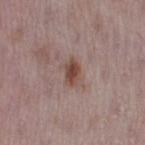{
  "biopsy_status": "not biopsied; imaged during a skin examination",
  "lesion_size": {
    "long_diameter_mm_approx": 3.0
  },
  "image": {
    "source": "total-body photography crop",
    "field_of_view_mm": 15
  },
  "patient": {
    "sex": "female",
    "age_approx": 30
  },
  "lighting": "white-light",
  "automated_metrics": {
    "color_variation_0_10": 4.0,
    "peripheral_color_asymmetry": 1.5
  },
  "site": "leg"
}